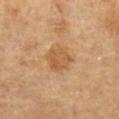Part of a total-body skin-imaging series; this lesion was reviewed on a skin check and was not flagged for biopsy. Cropped from a total-body skin-imaging series; the visible field is about 15 mm. A female subject, in their mid-60s. Approximately 3.5 mm at its widest. The lesion is on the left forearm. An algorithmic analysis of the crop reported an average lesion color of about L≈56 a*≈20 b*≈39 (CIELAB), roughly 8 lightness units darker than nearby skin, and a lesion-to-skin contrast of about 6 (normalized; higher = more distinct). The software also gave a border-irregularity rating of about 2/10, internal color variation of about 2.5 on a 0–10 scale, and radial color variation of about 1. The analysis additionally found a detector confidence of about 100 out of 100 that the crop contains a lesion. The tile uses cross-polarized illumination.